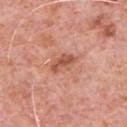The lesion was tiled from a total-body skin photograph and was not biopsied. The lesion is located on the chest. Imaged with white-light lighting. This image is a 15 mm lesion crop taken from a total-body photograph. A male patient, aged approximately 80.